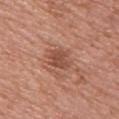A female subject about 50 years old.
A 15 mm crop from a total-body photograph taken for skin-cancer surveillance.
The lesion is located on the upper back.